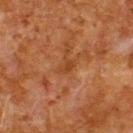workup: no biopsy performed (imaged during a skin exam) | body site: the upper back | imaging modality: ~15 mm crop, total-body skin-cancer survey | image-analysis metrics: roughly 5 lightness units darker than nearby skin and a normalized border contrast of about 5.5; a border-irregularity index near 3.5/10, internal color variation of about 0 on a 0–10 scale, and peripheral color asymmetry of about 0 | subject: male, aged approximately 80 | lesion diameter: ~2.5 mm (longest diameter).